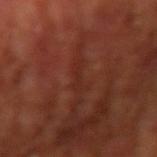The lesion was photographed on a routine skin check and not biopsied; there is no pathology result. A lesion tile, about 15 mm wide, cut from a 3D total-body photograph. The total-body-photography lesion software estimated a lesion area of about 2.5 mm², an eccentricity of roughly 0.95, and a shape-asymmetry score of about 0.4 (0 = symmetric). The analysis additionally found internal color variation of about 0 on a 0–10 scale and a peripheral color-asymmetry measure near 0. A male patient aged 63–67. From the right upper arm. This is a cross-polarized tile. Longest diameter approximately 2.5 mm.